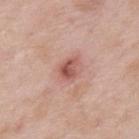The lesion was photographed on a routine skin check and not biopsied; there is no pathology result. The lesion is located on the upper back. A male subject, approximately 55 years of age. This image is a 15 mm lesion crop taken from a total-body photograph.